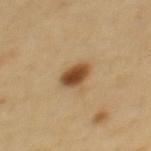{"biopsy_status": "not biopsied; imaged during a skin examination"}